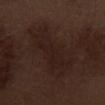follow-up=no biopsy performed (imaged during a skin exam) | tile lighting=white-light | image source=~15 mm tile from a whole-body skin photo | anatomic site=the abdomen | patient=male, about 70 years old.